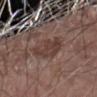notes = total-body-photography surveillance lesion; no biopsy | lesion size = about 4.5 mm | patient = male, about 80 years old | automated metrics = a lesion area of about 11 mm², an eccentricity of roughly 0.7, and two-axis asymmetry of about 0.25; a lesion color around L≈39 a*≈17 b*≈22 in CIELAB and roughly 8 lightness units darker than nearby skin; internal color variation of about 3 on a 0–10 scale and radial color variation of about 1 | tile lighting = white-light illumination | acquisition = ~15 mm crop, total-body skin-cancer survey | location = the right arm.